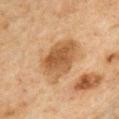<lesion>
<biopsy_status>not biopsied; imaged during a skin examination</biopsy_status>
<image>
  <source>total-body photography crop</source>
  <field_of_view_mm>15</field_of_view_mm>
</image>
<site>chest</site>
<automated_metrics>
  <area_mm2_approx>17.0</area_mm2_approx>
  <eccentricity>0.7</eccentricity>
  <shape_asymmetry>0.2</shape_asymmetry>
  <cielab_L>45</cielab_L>
  <cielab_a>17</cielab_a>
  <cielab_b>33</cielab_b>
  <vs_skin_darker_L>10.0</vs_skin_darker_L>
  <vs_skin_contrast_norm>8.0</vs_skin_contrast_norm>
  <border_irregularity_0_10>2.5</border_irregularity_0_10>
  <color_variation_0_10>4.0</color_variation_0_10>
  <peripheral_color_asymmetry>1.5</peripheral_color_asymmetry>
  <nevus_likeness_0_100>10</nevus_likeness_0_100>
  <lesion_detection_confidence_0_100>100</lesion_detection_confidence_0_100>
</automated_metrics>
<lighting>cross-polarized</lighting>
<patient>
  <sex>male</sex>
  <age_approx>65</age_approx>
</patient>
<lesion_size>
  <long_diameter_mm_approx>5.5</long_diameter_mm_approx>
</lesion_size>
</lesion>